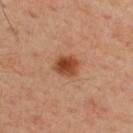No biopsy was performed on this lesion — it was imaged during a full skin examination and was not determined to be concerning. A male subject, aged approximately 55. The tile uses cross-polarized illumination. The lesion is on the upper back. A close-up tile cropped from a whole-body skin photograph, about 15 mm across. Automated tile analysis of the lesion measured a lesion area of about 6 mm² and an outline eccentricity of about 0.5 (0 = round, 1 = elongated). It also reported a border-irregularity index near 2/10 and a peripheral color-asymmetry measure near 1.5. About 3 mm across.